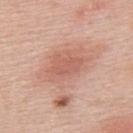{
  "biopsy_status": "not biopsied; imaged during a skin examination",
  "image": {
    "source": "total-body photography crop",
    "field_of_view_mm": 15
  },
  "lighting": "white-light",
  "automated_metrics": {
    "eccentricity": 0.85,
    "cielab_L": 59,
    "cielab_a": 26,
    "cielab_b": 29,
    "vs_skin_darker_L": 8.0,
    "border_irregularity_0_10": 3.5,
    "color_variation_0_10": 1.5,
    "peripheral_color_asymmetry": 0.5,
    "nevus_likeness_0_100": 70,
    "lesion_detection_confidence_0_100": 100
  },
  "site": "upper back",
  "lesion_size": {
    "long_diameter_mm_approx": 3.5
  },
  "patient": {
    "sex": "male",
    "age_approx": 55
  }
}